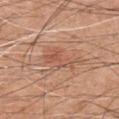Impression: This lesion was catalogued during total-body skin photography and was not selected for biopsy. Background: Imaged with white-light lighting. A 15 mm close-up tile from a total-body photography series done for melanoma screening. Located on the chest. The subject is a male aged 58–62.